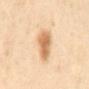Part of a total-body skin-imaging series; this lesion was reviewed on a skin check and was not flagged for biopsy. A region of skin cropped from a whole-body photographic capture, roughly 15 mm wide. Imaged with cross-polarized lighting. About 4.5 mm across. On the mid back. A male patient, aged approximately 65.